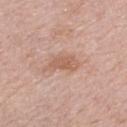follow-up: catalogued during a skin exam; not biopsied
illumination: white-light
location: the right upper arm
lesion diameter: about 4 mm
imaging modality: ~15 mm tile from a whole-body skin photo
patient: female, about 65 years old